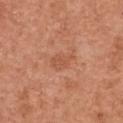| feature | finding |
|---|---|
| biopsy status | no biopsy performed (imaged during a skin exam) |
| location | the abdomen |
| acquisition | ~15 mm crop, total-body skin-cancer survey |
| lesion size | ≈3 mm |
| TBP lesion metrics | two-axis asymmetry of about 0.3; a lesion color around L≈54 a*≈26 b*≈34 in CIELAB, a lesion–skin lightness drop of about 7, and a normalized lesion–skin contrast near 5; a nevus-likeness score of about 0/100 and lesion-presence confidence of about 100/100 |
| patient | male, in their mid- to late 60s |
| tile lighting | white-light |